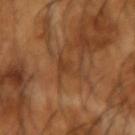| key | value |
|---|---|
| diameter | about 3.5 mm |
| lighting | cross-polarized |
| subject | male, aged approximately 65 |
| acquisition | ~15 mm tile from a whole-body skin photo |
| site | the left forearm |